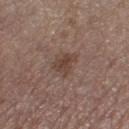Clinical impression:
Imaged during a routine full-body skin examination; the lesion was not biopsied and no histopathology is available.
Context:
The lesion is located on the left lower leg. Longest diameter approximately 3.5 mm. A roughly 15 mm field-of-view crop from a total-body skin photograph. The total-body-photography lesion software estimated a lesion color around L≈42 a*≈17 b*≈23 in CIELAB and about 8 CIELAB-L* units darker than the surrounding skin. The analysis additionally found a border-irregularity index near 4/10, a within-lesion color-variation index near 3/10, and radial color variation of about 1. And it measured a detector confidence of about 100 out of 100 that the crop contains a lesion. The subject is a female roughly 65 years of age.